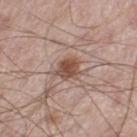Part of a total-body skin-imaging series; this lesion was reviewed on a skin check and was not flagged for biopsy. A lesion tile, about 15 mm wide, cut from a 3D total-body photograph. The tile uses white-light illumination. On the right thigh. The lesion-visualizer software estimated an average lesion color of about L≈50 a*≈20 b*≈26 (CIELAB), a lesion–skin lightness drop of about 12, and a lesion-to-skin contrast of about 9 (normalized; higher = more distinct). The analysis additionally found a border-irregularity rating of about 2.5/10. A male patient, aged 63–67. Approximately 3 mm at its widest.